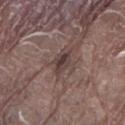The lesion is located on the left lower leg. Cropped from a whole-body photographic skin survey; the tile spans about 15 mm. The patient is a male about 80 years old. The tile uses white-light illumination.